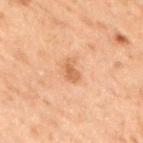Assessment:
Imaged during a routine full-body skin examination; the lesion was not biopsied and no histopathology is available.
Clinical summary:
The patient is a male aged approximately 70. The lesion is located on the right thigh. A 15 mm close-up extracted from a 3D total-body photography capture.